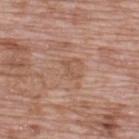biopsy status: total-body-photography surveillance lesion; no biopsy | patient: male, about 70 years old | acquisition: ~15 mm crop, total-body skin-cancer survey | body site: the upper back | tile lighting: white-light illumination | size: about 2.5 mm.